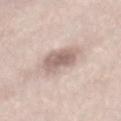No biopsy was performed on this lesion — it was imaged during a full skin examination and was not determined to be concerning. The lesion is on the back. Cropped from a total-body skin-imaging series; the visible field is about 15 mm. The subject is a male aged around 75. Imaged with white-light lighting. An algorithmic analysis of the crop reported an eccentricity of roughly 0.6. The software also gave a mean CIELAB color near L≈63 a*≈15 b*≈22, about 12 CIELAB-L* units darker than the surrounding skin, and a normalized border contrast of about 7.5. And it measured border irregularity of about 3.5 on a 0–10 scale. The software also gave a nevus-likeness score of about 35/100 and lesion-presence confidence of about 95/100.